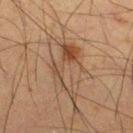Findings:
* biopsy status · no biopsy performed (imaged during a skin exam)
* subject · male, aged approximately 35
* diameter · ~7 mm (longest diameter)
* location · the right thigh
* image source · ~15 mm tile from a whole-body skin photo
* tile lighting · cross-polarized illumination